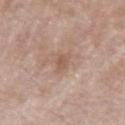follow-up: catalogued during a skin exam; not biopsied | body site: the left upper arm | subject: male, aged 53 to 57 | image source: total-body-photography crop, ~15 mm field of view | image-analysis metrics: a lesion–skin lightness drop of about 8; a nevus-likeness score of about 0/100 and a detector confidence of about 100 out of 100 that the crop contains a lesion.